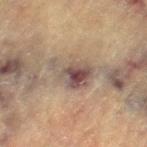The lesion was tiled from a total-body skin photograph and was not biopsied. A female subject, roughly 80 years of age. Approximately 5 mm at its widest. A lesion tile, about 15 mm wide, cut from a 3D total-body photograph. Automated tile analysis of the lesion measured a lesion color around L≈43 a*≈13 b*≈19 in CIELAB, roughly 10 lightness units darker than nearby skin, and a lesion-to-skin contrast of about 9.5 (normalized; higher = more distinct). Captured under cross-polarized illumination. The lesion is located on the left thigh.